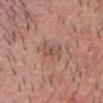| key | value |
|---|---|
| biopsy status | total-body-photography surveillance lesion; no biopsy |
| anatomic site | the head or neck |
| acquisition | total-body-photography crop, ~15 mm field of view |
| lighting | white-light illumination |
| diameter | about 3 mm |
| subject | male, in their 40s |